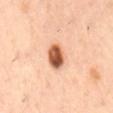{
  "biopsy_status": "not biopsied; imaged during a skin examination",
  "image": {
    "source": "total-body photography crop",
    "field_of_view_mm": 15
  },
  "patient": {
    "sex": "male",
    "age_approx": 55
  },
  "site": "mid back"
}